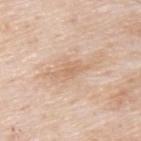Captured during whole-body skin photography for melanoma surveillance; the lesion was not biopsied. A male subject, aged 78 to 82. The recorded lesion diameter is about 4 mm. A roughly 15 mm field-of-view crop from a total-body skin photograph. Imaged with white-light lighting. The lesion is on the upper back. Automated tile analysis of the lesion measured a footprint of about 4.5 mm², a shape eccentricity near 0.9, and a symmetry-axis asymmetry near 0.4. And it measured a within-lesion color-variation index near 1.5/10 and peripheral color asymmetry of about 0.5. It also reported a lesion-detection confidence of about 100/100.